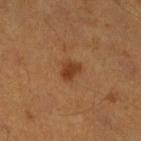Case summary:
– notes · no biopsy performed (imaged during a skin exam)
– anatomic site · the leg
– subject · male, about 55 years old
– illumination · cross-polarized
– image source · ~15 mm tile from a whole-body skin photo